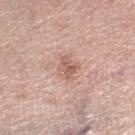Q: Was a biopsy performed?
A: total-body-photography surveillance lesion; no biopsy
Q: Who is the patient?
A: female, aged around 65
Q: What kind of image is this?
A: total-body-photography crop, ~15 mm field of view
Q: How large is the lesion?
A: about 3 mm
Q: Automated lesion metrics?
A: a mean CIELAB color near L≈60 a*≈21 b*≈26, roughly 10 lightness units darker than nearby skin, and a lesion-to-skin contrast of about 6.5 (normalized; higher = more distinct); a nevus-likeness score of about 5/100 and a lesion-detection confidence of about 100/100
Q: Where on the body is the lesion?
A: the right lower leg